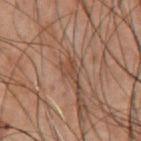• follow-up · catalogued during a skin exam; not biopsied
• subject · male, aged around 70
• image · 15 mm crop, total-body photography
• body site · the chest
• diameter · about 2.5 mm
• lighting · cross-polarized illumination